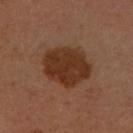Findings:
* workup: catalogued during a skin exam; not biopsied